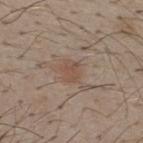Notes:
– notes — total-body-photography surveillance lesion; no biopsy
– lighting — white-light illumination
– patient — male, about 30 years old
– image — 15 mm crop, total-body photography
– lesion size — about 2.5 mm
– anatomic site — the upper back
– automated metrics — a footprint of about 4.5 mm² and two-axis asymmetry of about 0.2; roughly 6 lightness units darker than nearby skin and a lesion-to-skin contrast of about 5.5 (normalized; higher = more distinct); border irregularity of about 2 on a 0–10 scale, a color-variation rating of about 1.5/10, and peripheral color asymmetry of about 0.5